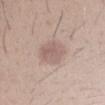notes = total-body-photography surveillance lesion; no biopsy
tile lighting = white-light
subject = male, about 30 years old
acquisition = 15 mm crop, total-body photography
automated lesion analysis = a mean CIELAB color near L≈59 a*≈16 b*≈22, roughly 9 lightness units darker than nearby skin, and a normalized lesion–skin contrast near 6; a border-irregularity index near 1.5/10
body site = the left forearm